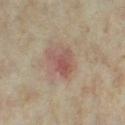{
  "site": "leg",
  "image": {
    "source": "total-body photography crop",
    "field_of_view_mm": 15
  },
  "patient": {
    "sex": "female",
    "age_approx": 35
  },
  "lesion_size": {
    "long_diameter_mm_approx": 3.5
  },
  "lighting": "cross-polarized"
}